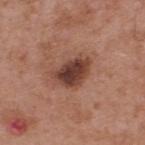Captured during whole-body skin photography for melanoma surveillance; the lesion was not biopsied.
The subject is a male in their mid- to late 50s.
On the upper back.
Cropped from a total-body skin-imaging series; the visible field is about 15 mm.
About 4.5 mm across.
Imaged with white-light lighting.